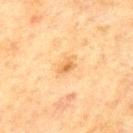Part of a total-body skin-imaging series; this lesion was reviewed on a skin check and was not flagged for biopsy.
Measured at roughly 2.5 mm in maximum diameter.
A male subject about 70 years old.
Imaged with cross-polarized lighting.
The lesion-visualizer software estimated a lesion area of about 3.5 mm², a shape eccentricity near 0.8, and a shape-asymmetry score of about 0.25 (0 = symmetric). The analysis additionally found border irregularity of about 2.5 on a 0–10 scale, a within-lesion color-variation index near 3/10, and radial color variation of about 1.
A region of skin cropped from a whole-body photographic capture, roughly 15 mm wide.
The lesion is located on the back.